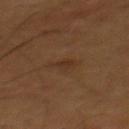A male subject in their 60s. This is a cross-polarized tile. The lesion-visualizer software estimated a lesion-to-skin contrast of about 5 (normalized; higher = more distinct). The analysis additionally found an automated nevus-likeness rating near 35 out of 100 and a detector confidence of about 100 out of 100 that the crop contains a lesion. A lesion tile, about 15 mm wide, cut from a 3D total-body photograph. Approximately 3 mm at its widest. On the mid back.